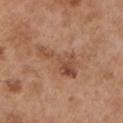Impression:
No biopsy was performed on this lesion — it was imaged during a full skin examination and was not determined to be concerning.
Context:
Automated tile analysis of the lesion measured a symmetry-axis asymmetry near 0.6. The software also gave an average lesion color of about L≈50 a*≈22 b*≈32 (CIELAB), roughly 9 lightness units darker than nearby skin, and a normalized border contrast of about 6.5. The software also gave a color-variation rating of about 5.5/10 and peripheral color asymmetry of about 2. The software also gave a classifier nevus-likeness of about 0/100 and a lesion-detection confidence of about 100/100. The lesion is located on the left upper arm. Approximately 6 mm at its widest. A male subject, roughly 55 years of age. Imaged with white-light lighting. A region of skin cropped from a whole-body photographic capture, roughly 15 mm wide.